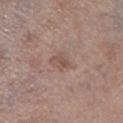Part of a total-body skin-imaging series; this lesion was reviewed on a skin check and was not flagged for biopsy.
The tile uses white-light illumination.
The patient is a male about 70 years old.
Located on the right lower leg.
The recorded lesion diameter is about 2.5 mm.
A roughly 15 mm field-of-view crop from a total-body skin photograph.
The total-body-photography lesion software estimated border irregularity of about 2.5 on a 0–10 scale.